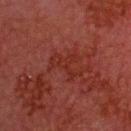Acquisition and patient details:
The total-body-photography lesion software estimated a footprint of about 7 mm², an eccentricity of roughly 0.7, and two-axis asymmetry of about 0.65. The analysis additionally found a mean CIELAB color near L≈23 a*≈24 b*≈23 and a lesion–skin lightness drop of about 4. The software also gave a detector confidence of about 85 out of 100 that the crop contains a lesion. A region of skin cropped from a whole-body photographic capture, roughly 15 mm wide. The recorded lesion diameter is about 4 mm. From the head or neck. A male patient in their 60s. Captured under cross-polarized illumination.